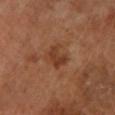No biopsy was performed on this lesion — it was imaged during a full skin examination and was not determined to be concerning.
Located on the left lower leg.
Cropped from a whole-body photographic skin survey; the tile spans about 15 mm.
The recorded lesion diameter is about 3 mm.
The subject is a female aged 63 to 67.
Imaged with cross-polarized lighting.